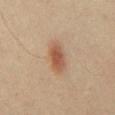biopsy_status: not biopsied; imaged during a skin examination
image:
  source: total-body photography crop
  field_of_view_mm: 15
patient:
  sex: male
  age_approx: 50
site: chest
lighting: cross-polarized
automated_metrics:
  area_mm2_approx: 7.0
  cielab_L: 48
  cielab_a: 19
  cielab_b: 28
  vs_skin_darker_L: 10.0
  vs_skin_contrast_norm: 8.0
  border_irregularity_0_10: 2.5
  color_variation_0_10: 3.5
  peripheral_color_asymmetry: 1.0